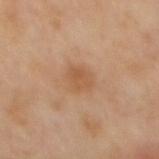Q: Was this lesion biopsied?
A: imaged on a skin check; not biopsied
Q: Where on the body is the lesion?
A: the mid back
Q: Automated lesion metrics?
A: a mean CIELAB color near L≈56 a*≈21 b*≈36 and about 7 CIELAB-L* units darker than the surrounding skin; a color-variation rating of about 2/10 and a peripheral color-asymmetry measure near 1; lesion-presence confidence of about 100/100
Q: How large is the lesion?
A: ≈3 mm
Q: What lighting was used for the tile?
A: cross-polarized illumination
Q: How was this image acquired?
A: total-body-photography crop, ~15 mm field of view